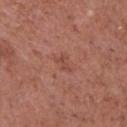The lesion was photographed on a routine skin check and not biopsied; there is no pathology result. On the head or neck. Cropped from a total-body skin-imaging series; the visible field is about 15 mm. Measured at roughly 2.5 mm in maximum diameter. Automated tile analysis of the lesion measured a nevus-likeness score of about 0/100 and a detector confidence of about 100 out of 100 that the crop contains a lesion. A male patient approximately 45 years of age. The tile uses white-light illumination.